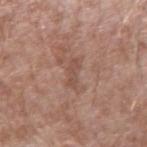Part of a total-body skin-imaging series; this lesion was reviewed on a skin check and was not flagged for biopsy.
A lesion tile, about 15 mm wide, cut from a 3D total-body photograph.
This is a white-light tile.
Approximately 3.5 mm at its widest.
An algorithmic analysis of the crop reported a footprint of about 3 mm², a shape eccentricity near 0.95, and two-axis asymmetry of about 0.3.
The subject is a male in their mid- to late 50s.
From the left upper arm.